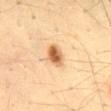Background:
Cropped from a total-body skin-imaging series; the visible field is about 15 mm. Captured under cross-polarized illumination. A male subject, aged around 35. Measured at roughly 3 mm in maximum diameter. From the mid back.